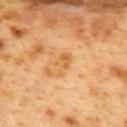Q: Was a biopsy performed?
A: catalogued during a skin exam; not biopsied
Q: Who is the patient?
A: female, aged around 40
Q: Where on the body is the lesion?
A: the upper back
Q: What is the lesion's diameter?
A: ≈3 mm
Q: What lighting was used for the tile?
A: cross-polarized
Q: What kind of image is this?
A: ~15 mm tile from a whole-body skin photo